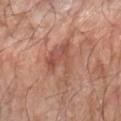The lesion was photographed on a routine skin check and not biopsied; there is no pathology result. The subject is a male roughly 60 years of age. The lesion's longest dimension is about 5.5 mm. A close-up tile cropped from a whole-body skin photograph, about 15 mm across. The lesion is on the left forearm.